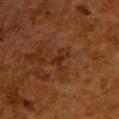Q: Is there a histopathology result?
A: total-body-photography surveillance lesion; no biopsy
Q: Who is the patient?
A: female, roughly 50 years of age
Q: What is the lesion's diameter?
A: ~2.5 mm (longest diameter)
Q: Where on the body is the lesion?
A: the upper back
Q: What kind of image is this?
A: ~15 mm crop, total-body skin-cancer survey
Q: Illumination type?
A: cross-polarized illumination